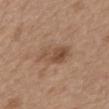{"biopsy_status": "not biopsied; imaged during a skin examination"}